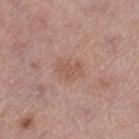Background: Automated tile analysis of the lesion measured border irregularity of about 3 on a 0–10 scale, a within-lesion color-variation index near 1.5/10, and a peripheral color-asymmetry measure near 0.5. This image is a 15 mm lesion crop taken from a total-body photograph. This is a white-light tile. The lesion is on the right lower leg. A female subject approximately 65 years of age.